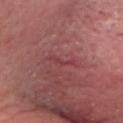The lesion was photographed on a routine skin check and not biopsied; there is no pathology result. Automated tile analysis of the lesion measured a shape eccentricity near 0.95 and two-axis asymmetry of about 0.5. And it measured roughly 6 lightness units darker than nearby skin and a normalized lesion–skin contrast near 5. The software also gave border irregularity of about 6 on a 0–10 scale, a color-variation rating of about 0/10, and a peripheral color-asymmetry measure near 0. Imaged with white-light lighting. A 15 mm close-up extracted from a 3D total-body photography capture. The lesion's longest dimension is about 3 mm. A male patient, in their mid-60s. Located on the head or neck.